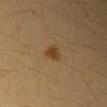The lesion was tiled from a total-body skin photograph and was not biopsied. The lesion is on the right upper arm. The patient is a female aged approximately 30. A 15 mm close-up tile from a total-body photography series done for melanoma screening.